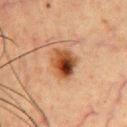<lesion>
  <image>
    <source>total-body photography crop</source>
    <field_of_view_mm>15</field_of_view_mm>
  </image>
  <lighting>cross-polarized</lighting>
  <site>chest</site>
  <automated_metrics>
    <border_irregularity_0_10>2.0</border_irregularity_0_10>
    <color_variation_0_10>10.0</color_variation_0_10>
    <peripheral_color_asymmetry>4.5</peripheral_color_asymmetry>
  </automated_metrics>
  <patient>
    <sex>male</sex>
    <age_approx>50</age_approx>
  </patient>
</lesion>